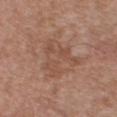| field | value |
|---|---|
| workup | catalogued during a skin exam; not biopsied |
| automated lesion analysis | a lesion color around L≈50 a*≈20 b*≈28 in CIELAB, about 6 CIELAB-L* units darker than the surrounding skin, and a normalized border contrast of about 5; border irregularity of about 8 on a 0–10 scale and peripheral color asymmetry of about 1 |
| patient | male, approximately 55 years of age |
| site | the chest |
| diameter | about 5.5 mm |
| image | total-body-photography crop, ~15 mm field of view |
| illumination | white-light illumination |